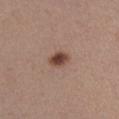Assessment: Captured during whole-body skin photography for melanoma surveillance; the lesion was not biopsied. Image and clinical context: The subject is a male about 55 years old. The tile uses white-light illumination. About 2.5 mm across. A 15 mm crop from a total-body photograph taken for skin-cancer surveillance. The lesion is located on the right upper arm.